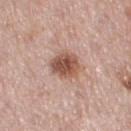Part of a total-body skin-imaging series; this lesion was reviewed on a skin check and was not flagged for biopsy.
Measured at roughly 4 mm in maximum diameter.
Cropped from a whole-body photographic skin survey; the tile spans about 15 mm.
On the right thigh.
A female patient, aged approximately 50.
Captured under white-light illumination.